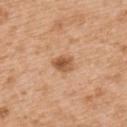follow-up: no biopsy performed (imaged during a skin exam) | lesion size: ~2.5 mm (longest diameter) | TBP lesion metrics: a lesion area of about 4.5 mm², a shape eccentricity near 0.6, and two-axis asymmetry of about 0.2; a mean CIELAB color near L≈56 a*≈23 b*≈36 and a lesion–skin lightness drop of about 12; a border-irregularity rating of about 2/10 and internal color variation of about 4.5 on a 0–10 scale | patient: male, aged 53 to 57 | image: 15 mm crop, total-body photography | location: the upper back | tile lighting: white-light.